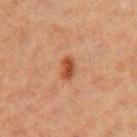Q: Patient demographics?
A: female, aged around 40
Q: How was the tile lit?
A: cross-polarized illumination
Q: What is the lesion's diameter?
A: ≈3 mm
Q: What is the imaging modality?
A: 15 mm crop, total-body photography
Q: What is the anatomic site?
A: the arm
Q: Automated lesion metrics?
A: a footprint of about 4.5 mm²; border irregularity of about 1.5 on a 0–10 scale, internal color variation of about 3 on a 0–10 scale, and peripheral color asymmetry of about 1; a nevus-likeness score of about 95/100